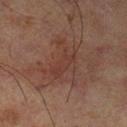| feature | finding |
|---|---|
| follow-up | no biopsy performed (imaged during a skin exam) |
| patient | male, in their 70s |
| acquisition | ~15 mm tile from a whole-body skin photo |
| anatomic site | the left lower leg |